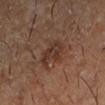No biopsy was performed on this lesion — it was imaged during a full skin examination and was not determined to be concerning.
Automated image analysis of the tile measured a lesion area of about 7.5 mm², an outline eccentricity of about 0.65 (0 = round, 1 = elongated), and a shape-asymmetry score of about 0.35 (0 = symmetric). It also reported an average lesion color of about L≈32 a*≈18 b*≈24 (CIELAB) and a lesion–skin lightness drop of about 7. The analysis additionally found border irregularity of about 4.5 on a 0–10 scale, a within-lesion color-variation index near 3.5/10, and peripheral color asymmetry of about 1.5. And it measured a nevus-likeness score of about 15/100.
A roughly 15 mm field-of-view crop from a total-body skin photograph.
The subject is a male approximately 55 years of age.
The lesion's longest dimension is about 3.5 mm.
The tile uses cross-polarized illumination.
Located on the left forearm.